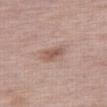{
  "biopsy_status": "not biopsied; imaged during a skin examination",
  "lighting": "white-light",
  "image": {
    "source": "total-body photography crop",
    "field_of_view_mm": 15
  },
  "lesion_size": {
    "long_diameter_mm_approx": 3.0
  },
  "site": "left leg",
  "automated_metrics": {
    "area_mm2_approx": 4.0,
    "eccentricity": 0.8,
    "shape_asymmetry": 0.35,
    "cielab_L": 55,
    "cielab_a": 20,
    "cielab_b": 24,
    "vs_skin_darker_L": 10.0,
    "vs_skin_contrast_norm": 7.0,
    "color_variation_0_10": 2.5,
    "peripheral_color_asymmetry": 1.0,
    "nevus_likeness_0_100": 40,
    "lesion_detection_confidence_0_100": 100
  },
  "patient": {
    "sex": "female",
    "age_approx": 65
  }
}